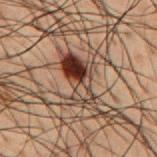{
  "biopsy_status": "not biopsied; imaged during a skin examination",
  "site": "mid back",
  "image": {
    "source": "total-body photography crop",
    "field_of_view_mm": 15
  },
  "lighting": "cross-polarized",
  "patient": {
    "sex": "male",
    "age_approx": 50
  },
  "lesion_size": {
    "long_diameter_mm_approx": 5.5
  }
}